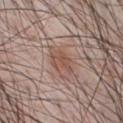Findings:
- biopsy status: no biopsy performed (imaged during a skin exam)
- image source: 15 mm crop, total-body photography
- patient: male, about 40 years old
- anatomic site: the chest
- tile lighting: white-light illumination